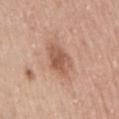Q: Was a biopsy performed?
A: catalogued during a skin exam; not biopsied
Q: How was the tile lit?
A: white-light illumination
Q: What is the lesion's diameter?
A: about 3.5 mm
Q: What kind of image is this?
A: ~15 mm crop, total-body skin-cancer survey
Q: Patient demographics?
A: female, aged around 60
Q: What did automated image analysis measure?
A: a lesion area of about 8.5 mm² and two-axis asymmetry of about 0.25; a border-irregularity rating of about 2.5/10, a color-variation rating of about 3.5/10, and a peripheral color-asymmetry measure near 1
Q: What is the anatomic site?
A: the right upper arm